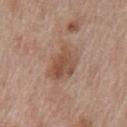Assessment:
Part of a total-body skin-imaging series; this lesion was reviewed on a skin check and was not flagged for biopsy.
Image and clinical context:
From the chest. A 15 mm close-up tile from a total-body photography series done for melanoma screening. A male subject aged 68–72.